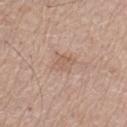biopsy status: imaged on a skin check; not biopsied | image-analysis metrics: a lesion area of about 4 mm², an eccentricity of roughly 0.75, and a symmetry-axis asymmetry near 0.2; a within-lesion color-variation index near 2/10 and radial color variation of about 1; a classifier nevus-likeness of about 0/100 | location: the leg | subject: male, roughly 65 years of age | image source: ~15 mm tile from a whole-body skin photo | lesion size: about 2.5 mm.